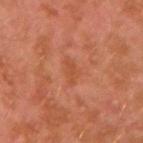Assessment: The lesion was tiled from a total-body skin photograph and was not biopsied. Background: Captured under cross-polarized illumination. The patient is a male about 30 years old. From the left arm. A close-up tile cropped from a whole-body skin photograph, about 15 mm across. Longest diameter approximately 3 mm.